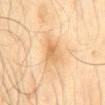Case summary:
• workup · no biopsy performed (imaged during a skin exam)
• acquisition · total-body-photography crop, ~15 mm field of view
• patient · male, aged approximately 65
• site · the mid back
• tile lighting · cross-polarized
• TBP lesion metrics · border irregularity of about 2 on a 0–10 scale, a color-variation rating of about 4/10, and peripheral color asymmetry of about 1.5; a classifier nevus-likeness of about 0/100
• lesion size · about 2.5 mm